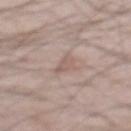<lesion>
  <biopsy_status>not biopsied; imaged during a skin examination</biopsy_status>
  <lesion_size>
    <long_diameter_mm_approx>3.5</long_diameter_mm_approx>
  </lesion_size>
  <lighting>white-light</lighting>
  <image>
    <source>total-body photography crop</source>
    <field_of_view_mm>15</field_of_view_mm>
  </image>
  <site>mid back</site>
  <patient>
    <sex>male</sex>
    <age_approx>65</age_approx>
  </patient>
</lesion>